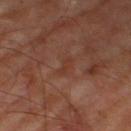Imaged during a routine full-body skin examination; the lesion was not biopsied and no histopathology is available.
This image is a 15 mm lesion crop taken from a total-body photograph.
Located on the right thigh.
A male subject aged 68 to 72.
The tile uses cross-polarized illumination.